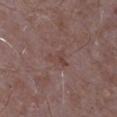Imaged during a routine full-body skin examination; the lesion was not biopsied and no histopathology is available.
From the arm.
A close-up tile cropped from a whole-body skin photograph, about 15 mm across.
The patient is a male roughly 65 years of age.
The lesion-visualizer software estimated a classifier nevus-likeness of about 0/100 and a lesion-detection confidence of about 100/100.